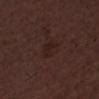Case summary:
- follow-up: total-body-photography surveillance lesion; no biopsy
- subject: male, about 50 years old
- lesion diameter: ≈2.5 mm
- tile lighting: white-light illumination
- acquisition: 15 mm crop, total-body photography